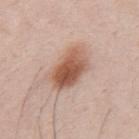Q: Is there a histopathology result?
A: imaged on a skin check; not biopsied
Q: What is the imaging modality?
A: 15 mm crop, total-body photography
Q: Where on the body is the lesion?
A: the back
Q: Who is the patient?
A: male, aged around 35